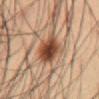This lesion was catalogued during total-body skin photography and was not selected for biopsy.